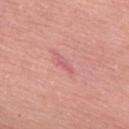Impression: The lesion was tiled from a total-body skin photograph and was not biopsied. Background: The lesion is located on the upper back. Automated image analysis of the tile measured an automated nevus-likeness rating near 0 out of 100 and lesion-presence confidence of about 60/100. Captured under white-light illumination. A close-up tile cropped from a whole-body skin photograph, about 15 mm across. About 2.5 mm across. A male subject, roughly 60 years of age.